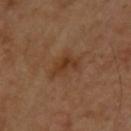Captured during whole-body skin photography for melanoma surveillance; the lesion was not biopsied.
Cropped from a whole-body photographic skin survey; the tile spans about 15 mm.
Located on the upper back.